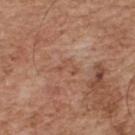biopsy status: catalogued during a skin exam; not biopsied
automated metrics: a footprint of about 3.5 mm² and a symmetry-axis asymmetry near 0.55; a nevus-likeness score of about 0/100 and a detector confidence of about 85 out of 100 that the crop contains a lesion
site: the upper back
subject: male, approximately 65 years of age
lesion size: about 3 mm
acquisition: 15 mm crop, total-body photography